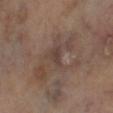biopsy_status: not biopsied; imaged during a skin examination
site: leg
image:
  source: total-body photography crop
  field_of_view_mm: 15
patient:
  sex: male
  age_approx: 70
automated_metrics:
  area_mm2_approx: 3.0
  eccentricity: 0.8
  shape_asymmetry: 0.45
  cielab_L: 38
  cielab_a: 15
  cielab_b: 20
  vs_skin_darker_L: 6.0
  vs_skin_contrast_norm: 6.0
  border_irregularity_0_10: 4.0
  nevus_likeness_0_100: 0
  lesion_detection_confidence_0_100: 70